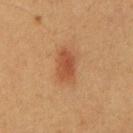• notes · no biopsy performed (imaged during a skin exam)
• subject · male, aged approximately 60
• image source · ~15 mm crop, total-body skin-cancer survey
• automated metrics · a within-lesion color-variation index near 3/10 and peripheral color asymmetry of about 1; a classifier nevus-likeness of about 100/100 and lesion-presence confidence of about 100/100
• lesion diameter · about 4 mm
• anatomic site · the chest